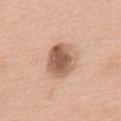The lesion was photographed on a routine skin check and not biopsied; there is no pathology result.
Imaged with white-light lighting.
Located on the back.
A male subject, approximately 75 years of age.
A roughly 15 mm field-of-view crop from a total-body skin photograph.
The lesion's longest dimension is about 4.5 mm.